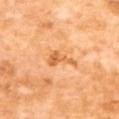The lesion was tiled from a total-body skin photograph and was not biopsied.
A female subject, aged approximately 55.
This is a cross-polarized tile.
Located on the upper back.
About 3.5 mm across.
A lesion tile, about 15 mm wide, cut from a 3D total-body photograph.
Automated image analysis of the tile measured an area of roughly 4 mm² and two-axis asymmetry of about 0.65. And it measured an average lesion color of about L≈66 a*≈29 b*≈47 (CIELAB) and about 11 CIELAB-L* units darker than the surrounding skin. And it measured a detector confidence of about 100 out of 100 that the crop contains a lesion.